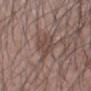{"biopsy_status": "not biopsied; imaged during a skin examination", "patient": {"sex": "male", "age_approx": 25}, "image": {"source": "total-body photography crop", "field_of_view_mm": 15}, "lesion_size": {"long_diameter_mm_approx": 4.0}, "lighting": "white-light", "automated_metrics": {"area_mm2_approx": 8.0, "eccentricity": 0.7, "shape_asymmetry": 0.25, "cielab_L": 46, "cielab_a": 16, "cielab_b": 22, "vs_skin_darker_L": 7.0, "color_variation_0_10": 3.0}, "site": "left forearm"}